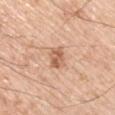Clinical impression:
This lesion was catalogued during total-body skin photography and was not selected for biopsy.
Acquisition and patient details:
Approximately 2.5 mm at its widest. Imaged with white-light lighting. Cropped from a total-body skin-imaging series; the visible field is about 15 mm. The subject is a male in their mid-50s. On the left upper arm.